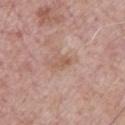automated_metrics:
  cielab_L: 57
  cielab_a: 20
  cielab_b: 28
  vs_skin_darker_L: 7.0
  vs_skin_contrast_norm: 5.5
  nevus_likeness_0_100: 0
  lesion_detection_confidence_0_100: 100
lesion_size:
  long_diameter_mm_approx: 2.5
lighting: white-light
patient:
  sex: male
  age_approx: 55
image:
  source: total-body photography crop
  field_of_view_mm: 15